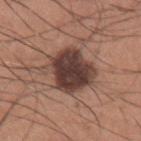workup: no biopsy performed (imaged during a skin exam)
anatomic site: the left upper arm
subject: male, in their mid-30s
illumination: white-light
size: ~5.5 mm (longest diameter)
acquisition: 15 mm crop, total-body photography